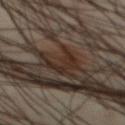Recorded during total-body skin imaging; not selected for excision or biopsy. A region of skin cropped from a whole-body photographic capture, roughly 15 mm wide. A male patient, approximately 45 years of age. On the abdomen.